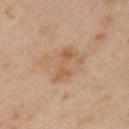• notes: catalogued during a skin exam; not biopsied
• diameter: ≈4 mm
• subject: female, aged approximately 40
• illumination: cross-polarized
• acquisition: ~15 mm tile from a whole-body skin photo
• anatomic site: the left upper arm